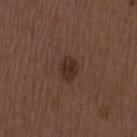follow-up=total-body-photography surveillance lesion; no biopsy
patient=male, roughly 50 years of age
diameter=about 2.5 mm
image=15 mm crop, total-body photography